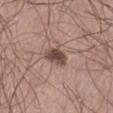Q: How was this image acquired?
A: total-body-photography crop, ~15 mm field of view
Q: Lesion location?
A: the left thigh
Q: Who is the patient?
A: male, in their mid-20s